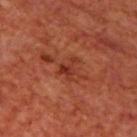Imaged during a routine full-body skin examination; the lesion was not biopsied and no histopathology is available.
A 15 mm close-up tile from a total-body photography series done for melanoma screening.
A male subject roughly 70 years of age.
The lesion is located on the upper back.